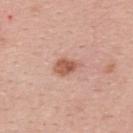biopsy status=total-body-photography surveillance lesion; no biopsy | subject=female, about 40 years old | lesion diameter=~3.5 mm (longest diameter) | site=the upper back | imaging modality=~15 mm crop, total-body skin-cancer survey | illumination=white-light.